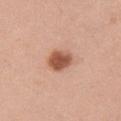The lesion is located on the right upper arm.
The subject is a female in their mid- to late 30s.
An algorithmic analysis of the crop reported a within-lesion color-variation index near 3.5/10 and peripheral color asymmetry of about 1. The software also gave a nevus-likeness score of about 100/100 and lesion-presence confidence of about 100/100.
A 15 mm close-up extracted from a 3D total-body photography capture.
Captured under white-light illumination.